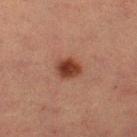biopsy_status: not biopsied; imaged during a skin examination
automated_metrics:
  area_mm2_approx: 6.5
  eccentricity: 0.55
  shape_asymmetry: 0.2
  border_irregularity_0_10: 2.0
  color_variation_0_10: 3.5
patient:
  sex: female
  age_approx: 55
site: right thigh
lighting: cross-polarized
image:
  source: total-body photography crop
  field_of_view_mm: 15
lesion_size:
  long_diameter_mm_approx: 3.0